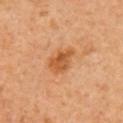Imaged during a routine full-body skin examination; the lesion was not biopsied and no histopathology is available.
The tile uses cross-polarized illumination.
From the right upper arm.
A male subject, in their 60s.
This image is a 15 mm lesion crop taken from a total-body photograph.
Automated tile analysis of the lesion measured two-axis asymmetry of about 0.25. It also reported a lesion color around L≈54 a*≈25 b*≈42 in CIELAB, a lesion–skin lightness drop of about 10, and a lesion-to-skin contrast of about 7.5 (normalized; higher = more distinct).